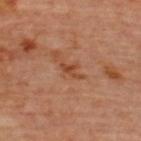Captured during whole-body skin photography for melanoma surveillance; the lesion was not biopsied.
The recorded lesion diameter is about 3 mm.
The tile uses cross-polarized illumination.
A close-up tile cropped from a whole-body skin photograph, about 15 mm across.
The lesion is on the upper back.
A female subject aged 43 to 47.